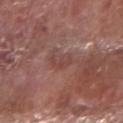Assessment: Imaged during a routine full-body skin examination; the lesion was not biopsied and no histopathology is available. Acquisition and patient details: From the right forearm. A 15 mm crop from a total-body photograph taken for skin-cancer surveillance. A male patient, aged approximately 80.